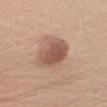Findings:
* follow-up — catalogued during a skin exam; not biopsied
* patient — female, in their 30s
* image source — total-body-photography crop, ~15 mm field of view
* location — the right upper arm